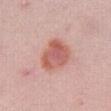notes: catalogued during a skin exam; not biopsied
site: the right thigh
subject: male, about 40 years old
TBP lesion metrics: an area of roughly 13 mm² and a shape-asymmetry score of about 0.2 (0 = symmetric); a lesion color around L≈59 a*≈27 b*≈27 in CIELAB, about 11 CIELAB-L* units darker than the surrounding skin, and a lesion-to-skin contrast of about 7.5 (normalized; higher = more distinct); internal color variation of about 4.5 on a 0–10 scale and radial color variation of about 1.5; a nevus-likeness score of about 100/100
acquisition: 15 mm crop, total-body photography
lesion diameter: about 4.5 mm
tile lighting: white-light illumination